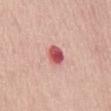{
  "biopsy_status": "not biopsied; imaged during a skin examination",
  "patient": {
    "sex": "female",
    "age_approx": 65
  },
  "automated_metrics": {
    "area_mm2_approx": 4.5,
    "shape_asymmetry": 0.1,
    "cielab_L": 55,
    "cielab_a": 35,
    "cielab_b": 24,
    "vs_skin_contrast_norm": 11.0,
    "border_irregularity_0_10": 1.0,
    "color_variation_0_10": 5.0,
    "peripheral_color_asymmetry": 1.5,
    "nevus_likeness_0_100": 0
  },
  "site": "mid back",
  "image": {
    "source": "total-body photography crop",
    "field_of_view_mm": 15
  },
  "lighting": "white-light"
}